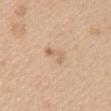Findings:
• notes: imaged on a skin check; not biopsied
• anatomic site: the arm
• subject: female, roughly 25 years of age
• illumination: white-light
• automated lesion analysis: a lesion-detection confidence of about 100/100
• lesion size: about 2.5 mm
• imaging modality: total-body-photography crop, ~15 mm field of view